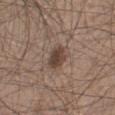notes: catalogued during a skin exam; not biopsied | tile lighting: white-light illumination | body site: the leg | image source: ~15 mm crop, total-body skin-cancer survey | TBP lesion metrics: an eccentricity of roughly 0.55 and a shape-asymmetry score of about 0.15 (0 = symmetric) | lesion diameter: about 3 mm | patient: male, in their mid-40s.